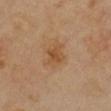biopsy status: total-body-photography surveillance lesion; no biopsy
patient: female, aged approximately 35
location: the chest
illumination: cross-polarized illumination
TBP lesion metrics: an average lesion color of about L≈48 a*≈19 b*≈35 (CIELAB) and a normalized lesion–skin contrast near 6.5; a border-irregularity index near 3/10, a within-lesion color-variation index near 2.5/10, and peripheral color asymmetry of about 1
image: ~15 mm crop, total-body skin-cancer survey
size: ≈2.5 mm